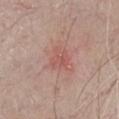Assessment:
Part of a total-body skin-imaging series; this lesion was reviewed on a skin check and was not flagged for biopsy.
Context:
Imaged with white-light lighting. The lesion's longest dimension is about 3 mm. A male patient in their 70s. Cropped from a whole-body photographic skin survey; the tile spans about 15 mm. From the chest.